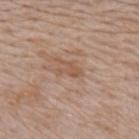Case summary:
- notes — imaged on a skin check; not biopsied
- acquisition — ~15 mm tile from a whole-body skin photo
- subject — male, about 65 years old
- diameter — ~3 mm (longest diameter)
- image-analysis metrics — a mean CIELAB color near L≈54 a*≈18 b*≈30 and about 7 CIELAB-L* units darker than the surrounding skin; border irregularity of about 6 on a 0–10 scale, a color-variation rating of about 1/10, and a peripheral color-asymmetry measure near 0.5; an automated nevus-likeness rating near 0 out of 100 and a lesion-detection confidence of about 100/100
- body site — the mid back